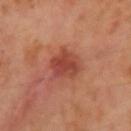biopsy_status: not biopsied; imaged during a skin examination
image:
  source: total-body photography crop
  field_of_view_mm: 15
patient:
  sex: female
  age_approx: 55
site: right upper arm